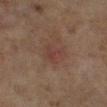| key | value |
|---|---|
| follow-up | imaged on a skin check; not biopsied |
| site | the left lower leg |
| image | ~15 mm tile from a whole-body skin photo |
| lighting | cross-polarized illumination |
| patient | female, in their 60s |
| diameter | about 3 mm |